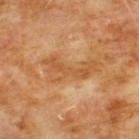{
  "biopsy_status": "not biopsied; imaged during a skin examination",
  "lighting": "cross-polarized",
  "site": "chest",
  "image": {
    "source": "total-body photography crop",
    "field_of_view_mm": 15
  },
  "lesion_size": {
    "long_diameter_mm_approx": 6.0
  },
  "patient": {
    "sex": "male",
    "age_approx": 60
  },
  "automated_metrics": {
    "cielab_L": 53,
    "cielab_a": 23,
    "cielab_b": 40,
    "vs_skin_darker_L": 7.0,
    "vs_skin_contrast_norm": 5.5,
    "nevus_likeness_0_100": 0,
    "lesion_detection_confidence_0_100": 95
  }
}